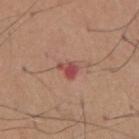Background: The lesion is on the chest. Cropped from a whole-body photographic skin survey; the tile spans about 15 mm. The patient is a male in their mid- to late 60s.